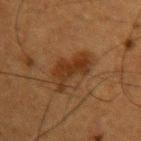notes: no biopsy performed (imaged during a skin exam); patient: male, aged 48–52; image: ~15 mm crop, total-body skin-cancer survey; site: the arm; diameter: about 5.5 mm.